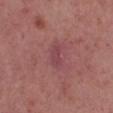Part of a total-body skin-imaging series; this lesion was reviewed on a skin check and was not flagged for biopsy. Located on the left lower leg. A roughly 15 mm field-of-view crop from a total-body skin photograph. A female patient aged 38 to 42.